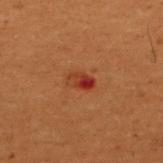This lesion was catalogued during total-body skin photography and was not selected for biopsy. On the upper back. The patient is a male aged 48–52. A roughly 15 mm field-of-view crop from a total-body skin photograph.